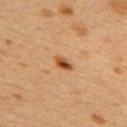Assessment: Part of a total-body skin-imaging series; this lesion was reviewed on a skin check and was not flagged for biopsy. Acquisition and patient details: Automated tile analysis of the lesion measured a classifier nevus-likeness of about 95/100 and a detector confidence of about 100 out of 100 that the crop contains a lesion. Longest diameter approximately 2.5 mm. The lesion is on the upper back. A lesion tile, about 15 mm wide, cut from a 3D total-body photograph. Captured under cross-polarized illumination. A female patient, in their 40s.